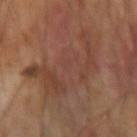Assessment: No biopsy was performed on this lesion — it was imaged during a full skin examination and was not determined to be concerning. Image and clinical context: A 15 mm crop from a total-body photograph taken for skin-cancer surveillance. The tile uses cross-polarized illumination. Measured at roughly 11 mm in maximum diameter. Located on the right forearm.